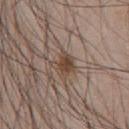Q: Was this lesion biopsied?
A: total-body-photography surveillance lesion; no biopsy
Q: What are the patient's age and sex?
A: male, aged 43–47
Q: How large is the lesion?
A: ≈3 mm
Q: What lighting was used for the tile?
A: white-light illumination
Q: What is the anatomic site?
A: the chest
Q: What is the imaging modality?
A: 15 mm crop, total-body photography
Q: Automated lesion metrics?
A: a shape eccentricity near 0.35 and two-axis asymmetry of about 0.3; a mean CIELAB color near L≈44 a*≈15 b*≈25, roughly 10 lightness units darker than nearby skin, and a normalized lesion–skin contrast near 8